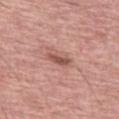* lesion size: ≈3 mm
* anatomic site: the left thigh
* lighting: white-light
* subject: female, about 60 years old
* image: total-body-photography crop, ~15 mm field of view